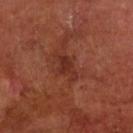Recorded during total-body skin imaging; not selected for excision or biopsy.
Located on the front of the torso.
Cropped from a total-body skin-imaging series; the visible field is about 15 mm.
The lesion-visualizer software estimated roughly 7 lightness units darker than nearby skin and a normalized lesion–skin contrast near 7. It also reported a border-irregularity rating of about 3.5/10 and radial color variation of about 0.5.
A male patient, about 70 years old.
Measured at roughly 3.5 mm in maximum diameter.